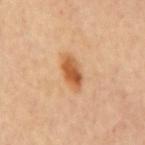biopsy_status: not biopsied; imaged during a skin examination
image:
  source: total-body photography crop
  field_of_view_mm: 15
lesion_size:
  long_diameter_mm_approx: 4.0
lighting: cross-polarized
site: back